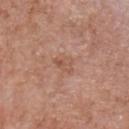Case summary:
– biopsy status · catalogued during a skin exam; not biopsied
– automated lesion analysis · a lesion color around L≈54 a*≈21 b*≈30 in CIELAB, about 7 CIELAB-L* units darker than the surrounding skin, and a lesion-to-skin contrast of about 5.5 (normalized; higher = more distinct); a classifier nevus-likeness of about 0/100
– acquisition · 15 mm crop, total-body photography
– patient · male, aged 58–62
– lesion diameter · about 2.5 mm
– anatomic site · the chest